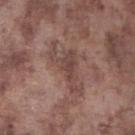No biopsy was performed on this lesion — it was imaged during a full skin examination and was not determined to be concerning.
A lesion tile, about 15 mm wide, cut from a 3D total-body photograph.
Imaged with white-light lighting.
On the left lower leg.
Approximately 6 mm at its widest.
A male patient aged approximately 75.
An algorithmic analysis of the crop reported a lesion area of about 10 mm², an outline eccentricity of about 0.9 (0 = round, 1 = elongated), and a symmetry-axis asymmetry near 0.65. The analysis additionally found a nevus-likeness score of about 0/100 and a lesion-detection confidence of about 75/100.